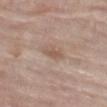follow-up = total-body-photography surveillance lesion; no biopsy.